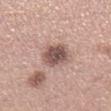Clinical impression: Part of a total-body skin-imaging series; this lesion was reviewed on a skin check and was not flagged for biopsy. Clinical summary: Captured under white-light illumination. The lesion is located on the right lower leg. The lesion-visualizer software estimated a footprint of about 8.5 mm², an eccentricity of roughly 0.35, and a shape-asymmetry score of about 0.15 (0 = symmetric). And it measured a mean CIELAB color near L≈52 a*≈19 b*≈21, roughly 15 lightness units darker than nearby skin, and a lesion-to-skin contrast of about 10 (normalized; higher = more distinct). The analysis additionally found a nevus-likeness score of about 5/100 and lesion-presence confidence of about 100/100. The patient is a female aged approximately 30. A roughly 15 mm field-of-view crop from a total-body skin photograph.